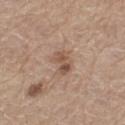Captured under white-light illumination. On the left thigh. A male subject approximately 65 years of age. About 3.5 mm across. A region of skin cropped from a whole-body photographic capture, roughly 15 mm wide.